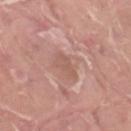biopsy status=no biopsy performed (imaged during a skin exam)
location=the left thigh
patient=male, roughly 40 years of age
acquisition=15 mm crop, total-body photography